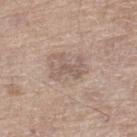Clinical impression: No biopsy was performed on this lesion — it was imaged during a full skin examination and was not determined to be concerning. Context: Imaged with white-light lighting. A male patient in their 70s. Located on the leg. Measured at roughly 3.5 mm in maximum diameter. Cropped from a total-body skin-imaging series; the visible field is about 15 mm.